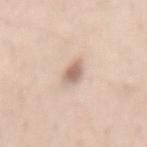No biopsy was performed on this lesion — it was imaged during a full skin examination and was not determined to be concerning. The tile uses white-light illumination. A roughly 15 mm field-of-view crop from a total-body skin photograph. The lesion-visualizer software estimated a footprint of about 4.5 mm², an eccentricity of roughly 0.8, and two-axis asymmetry of about 0.25. It also reported roughly 13 lightness units darker than nearby skin. And it measured border irregularity of about 2.5 on a 0–10 scale and internal color variation of about 3 on a 0–10 scale. The software also gave a classifier nevus-likeness of about 95/100. A female patient aged around 40. Measured at roughly 3 mm in maximum diameter. Located on the mid back.